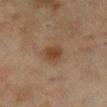Imaged during a routine full-body skin examination; the lesion was not biopsied and no histopathology is available. Cropped from a whole-body photographic skin survey; the tile spans about 15 mm. On the right lower leg. Measured at roughly 2.5 mm in maximum diameter. A female subject, in their mid- to late 50s.